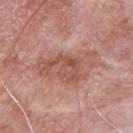Assessment: Part of a total-body skin-imaging series; this lesion was reviewed on a skin check and was not flagged for biopsy. Image and clinical context: Automated image analysis of the tile measured a mean CIELAB color near L≈54 a*≈23 b*≈28, about 9 CIELAB-L* units darker than the surrounding skin, and a normalized lesion–skin contrast near 6.5. It also reported border irregularity of about 6 on a 0–10 scale, a color-variation rating of about 5/10, and radial color variation of about 2. A male subject, approximately 65 years of age. A 15 mm crop from a total-body photograph taken for skin-cancer surveillance. Imaged with white-light lighting. The lesion is located on the chest.